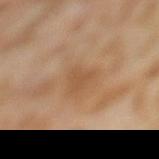workup: catalogued during a skin exam; not biopsied
acquisition: 15 mm crop, total-body photography
body site: the left thigh
patient: female, aged around 55
automated metrics: a mean CIELAB color near L≈54 a*≈19 b*≈36, a lesion–skin lightness drop of about 6, and a lesion-to-skin contrast of about 5 (normalized; higher = more distinct); a border-irregularity rating of about 4/10; a classifier nevus-likeness of about 0/100 and a detector confidence of about 100 out of 100 that the crop contains a lesion
illumination: cross-polarized illumination